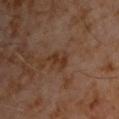Q: Was a biopsy performed?
A: imaged on a skin check; not biopsied
Q: Where on the body is the lesion?
A: the chest
Q: What kind of image is this?
A: ~15 mm tile from a whole-body skin photo
Q: What did automated image analysis measure?
A: a lesion area of about 2.5 mm²; about 7 CIELAB-L* units darker than the surrounding skin and a lesion-to-skin contrast of about 8 (normalized; higher = more distinct); an automated nevus-likeness rating near 0 out of 100
Q: What are the patient's age and sex?
A: male, aged 58 to 62